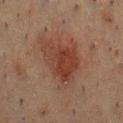Impression:
Imaged during a routine full-body skin examination; the lesion was not biopsied and no histopathology is available.
Clinical summary:
About 6.5 mm across. A male subject, in their 50s. The lesion is located on the chest. A 15 mm crop from a total-body photograph taken for skin-cancer surveillance. This is a cross-polarized tile.